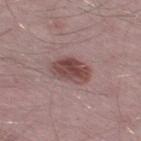Recorded during total-body skin imaging; not selected for excision or biopsy.
A male patient, aged around 50.
From the right thigh.
This image is a 15 mm lesion crop taken from a total-body photograph.